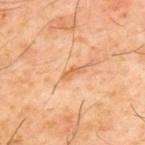| key | value |
|---|---|
| notes | total-body-photography surveillance lesion; no biopsy |
| lesion size | ~3 mm (longest diameter) |
| acquisition | ~15 mm crop, total-body skin-cancer survey |
| automated lesion analysis | a classifier nevus-likeness of about 0/100 |
| patient | male, aged 58 to 62 |
| anatomic site | the upper back |
| tile lighting | cross-polarized illumination |